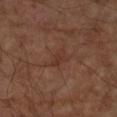Image and clinical context:
A male patient about 65 years old. The lesion is on the arm. A lesion tile, about 15 mm wide, cut from a 3D total-body photograph. The total-body-photography lesion software estimated a footprint of about 3.5 mm², a shape eccentricity near 0.8, and two-axis asymmetry of about 0.35. This is a cross-polarized tile.